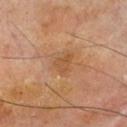Findings:
- image-analysis metrics · an area of roughly 4 mm² and a shape eccentricity near 0.7; a border-irregularity rating of about 3/10, internal color variation of about 1.5 on a 0–10 scale, and a peripheral color-asymmetry measure near 0.5; a nevus-likeness score of about 0/100 and a lesion-detection confidence of about 100/100
- tile lighting · cross-polarized illumination
- patient · male, roughly 70 years of age
- acquisition · ~15 mm crop, total-body skin-cancer survey
- body site · the chest